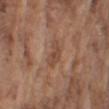{"biopsy_status": "not biopsied; imaged during a skin examination", "image": {"source": "total-body photography crop", "field_of_view_mm": 15}, "lighting": "white-light", "patient": {"sex": "male", "age_approx": 75}, "site": "right upper arm"}